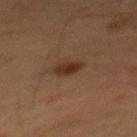follow-up: no biopsy performed (imaged during a skin exam) | subject: male, aged 63–67 | imaging modality: total-body-photography crop, ~15 mm field of view | location: the mid back.